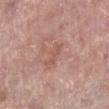This lesion was catalogued during total-body skin photography and was not selected for biopsy.
The total-body-photography lesion software estimated a mean CIELAB color near L≈55 a*≈23 b*≈25, roughly 6 lightness units darker than nearby skin, and a normalized lesion–skin contrast near 4.5. And it measured a border-irregularity index near 4.5/10 and radial color variation of about 0.
The lesion is on the right lower leg.
Captured under white-light illumination.
Longest diameter approximately 3.5 mm.
Cropped from a whole-body photographic skin survey; the tile spans about 15 mm.
A female patient aged approximately 60.